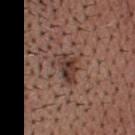The lesion was photographed on a routine skin check and not biopsied; there is no pathology result.
A lesion tile, about 15 mm wide, cut from a 3D total-body photograph.
About 4 mm across.
From the head or neck.
A male subject approximately 70 years of age.